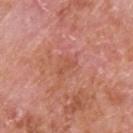Impression: The lesion was photographed on a routine skin check and not biopsied; there is no pathology result. Clinical summary: Located on the upper back. A male patient, about 65 years old. A 15 mm close-up tile from a total-body photography series done for melanoma screening.